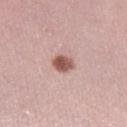Captured during whole-body skin photography for melanoma surveillance; the lesion was not biopsied. Automated tile analysis of the lesion measured a lesion area of about 5 mm², an outline eccentricity of about 0.5 (0 = round, 1 = elongated), and a shape-asymmetry score of about 0.2 (0 = symmetric). A close-up tile cropped from a whole-body skin photograph, about 15 mm across. Measured at roughly 2.5 mm in maximum diameter. Imaged with white-light lighting. A female subject roughly 35 years of age. On the right lower leg.